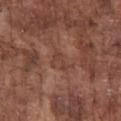Assessment:
Recorded during total-body skin imaging; not selected for excision or biopsy.
Context:
This is a white-light tile. Measured at roughly 3 mm in maximum diameter. The subject is a male approximately 75 years of age. Located on the chest. A close-up tile cropped from a whole-body skin photograph, about 15 mm across. Automated image analysis of the tile measured an area of roughly 3 mm², a shape eccentricity near 0.9, and a shape-asymmetry score of about 0.45 (0 = symmetric). The analysis additionally found border irregularity of about 5.5 on a 0–10 scale, a within-lesion color-variation index near 0.5/10, and peripheral color asymmetry of about 0.